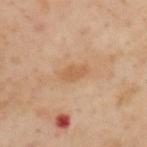{
  "biopsy_status": "not biopsied; imaged during a skin examination",
  "site": "upper back",
  "image": {
    "source": "total-body photography crop",
    "field_of_view_mm": 15
  },
  "lesion_size": {
    "long_diameter_mm_approx": 3.5
  },
  "patient": {
    "sex": "male",
    "age_approx": 55
  },
  "lighting": "cross-polarized"
}